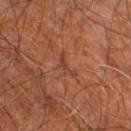Captured during whole-body skin photography for melanoma surveillance; the lesion was not biopsied. Longest diameter approximately 3 mm. The subject is a male aged 58–62. A close-up tile cropped from a whole-body skin photograph, about 15 mm across. From the right leg. Captured under cross-polarized illumination.